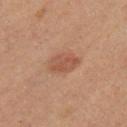Clinical impression:
The lesion was tiled from a total-body skin photograph and was not biopsied.
Clinical summary:
The lesion is located on the right thigh. A roughly 15 mm field-of-view crop from a total-body skin photograph. A female patient approximately 55 years of age.